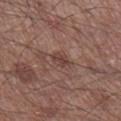Impression:
This lesion was catalogued during total-body skin photography and was not selected for biopsy.
Context:
A 15 mm close-up tile from a total-body photography series done for melanoma screening. The patient is a male roughly 60 years of age. The lesion is on the right lower leg. Approximately 2.5 mm at its widest. This is a white-light tile.